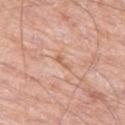biopsy status = total-body-photography surveillance lesion; no biopsy | site = the left thigh | patient = male, approximately 80 years of age | acquisition = 15 mm crop, total-body photography.